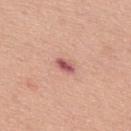notes: imaged on a skin check; not biopsied | image-analysis metrics: a footprint of about 3 mm² and an outline eccentricity of about 0.8 (0 = round, 1 = elongated); an average lesion color of about L≈56 a*≈28 b*≈24 (CIELAB) and roughly 14 lightness units darker than nearby skin; a lesion-detection confidence of about 100/100 | patient: male, in their mid- to late 40s | site: the upper back | lighting: white-light | image: 15 mm crop, total-body photography.